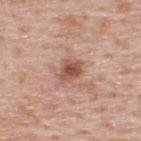Findings:
– follow-up: catalogued during a skin exam; not biopsied
– acquisition: ~15 mm tile from a whole-body skin photo
– anatomic site: the upper back
– tile lighting: white-light
– patient: male, aged 73 to 77
– automated lesion analysis: a footprint of about 6 mm², an eccentricity of roughly 0.7, and a shape-asymmetry score of about 0.25 (0 = symmetric); a lesion–skin lightness drop of about 12 and a normalized lesion–skin contrast near 8; border irregularity of about 2.5 on a 0–10 scale and peripheral color asymmetry of about 1